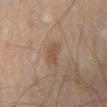notes: catalogued during a skin exam; not biopsied
size: ≈3 mm
location: the left lower leg
illumination: cross-polarized
patient: male, in their mid-40s
image source: 15 mm crop, total-body photography